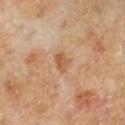Image and clinical context:
Cropped from a whole-body photographic skin survey; the tile spans about 15 mm. Automated tile analysis of the lesion measured a peripheral color-asymmetry measure near 1. Captured under cross-polarized illumination. A male patient approximately 65 years of age. The lesion is located on the leg.